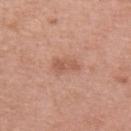The lesion was photographed on a routine skin check and not biopsied; there is no pathology result.
A female subject roughly 45 years of age.
A lesion tile, about 15 mm wide, cut from a 3D total-body photograph.
The total-body-photography lesion software estimated an area of roughly 3.5 mm² and an outline eccentricity of about 0.9 (0 = round, 1 = elongated). It also reported an average lesion color of about L≈56 a*≈24 b*≈30 (CIELAB) and roughly 8 lightness units darker than nearby skin. It also reported an automated nevus-likeness rating near 15 out of 100.
Measured at roughly 3 mm in maximum diameter.
From the left upper arm.